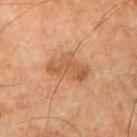Imaged during a routine full-body skin examination; the lesion was not biopsied and no histopathology is available.